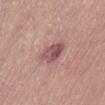Assessment:
Imaged during a routine full-body skin examination; the lesion was not biopsied and no histopathology is available.
Acquisition and patient details:
Measured at roughly 3.5 mm in maximum diameter. The patient is a male aged 38–42. Automated image analysis of the tile measured an eccentricity of roughly 0.7 and two-axis asymmetry of about 0.2. The software also gave a mean CIELAB color near L≈52 a*≈23 b*≈19, about 11 CIELAB-L* units darker than the surrounding skin, and a normalized border contrast of about 8. And it measured border irregularity of about 2 on a 0–10 scale. Cropped from a total-body skin-imaging series; the visible field is about 15 mm. This is a white-light tile. On the abdomen.